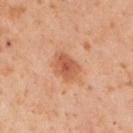Notes:
– image · 15 mm crop, total-body photography
– size · ~3 mm (longest diameter)
– subject · male, roughly 50 years of age
– tile lighting · cross-polarized
– location · the left upper arm